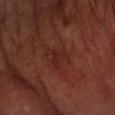No biopsy was performed on this lesion — it was imaged during a full skin examination and was not determined to be concerning. The patient is a male in their 70s. A lesion tile, about 15 mm wide, cut from a 3D total-body photograph. Located on the left forearm. About 3.5 mm across.